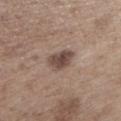Clinical impression:
The lesion was tiled from a total-body skin photograph and was not biopsied.
Image and clinical context:
A 15 mm close-up extracted from a 3D total-body photography capture. On the left lower leg. This is a white-light tile. A male subject, approximately 70 years of age. Longest diameter approximately 3.5 mm.